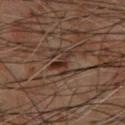workup: total-body-photography surveillance lesion; no biopsy
illumination: cross-polarized
subject: male, aged 58–62
location: the upper back
acquisition: ~15 mm tile from a whole-body skin photo
TBP lesion metrics: a mean CIELAB color near L≈27 a*≈16 b*≈22 and a lesion-to-skin contrast of about 8.5 (normalized; higher = more distinct); internal color variation of about 1.5 on a 0–10 scale and a peripheral color-asymmetry measure near 0.5; a detector confidence of about 85 out of 100 that the crop contains a lesion
lesion size: ~3.5 mm (longest diameter)